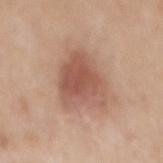Impression:
Recorded during total-body skin imaging; not selected for excision or biopsy.
Clinical summary:
The lesion is located on the back. About 6 mm across. This image is a 15 mm lesion crop taken from a total-body photograph. The tile uses white-light illumination. A female subject approximately 40 years of age.